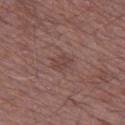This lesion was catalogued during total-body skin photography and was not selected for biopsy. A male patient roughly 50 years of age. The lesion is on the left thigh. An algorithmic analysis of the crop reported an average lesion color of about L≈43 a*≈19 b*≈21 (CIELAB), roughly 6 lightness units darker than nearby skin, and a normalized lesion–skin contrast near 5. The analysis additionally found a classifier nevus-likeness of about 0/100. About 2.5 mm across. Captured under white-light illumination. A roughly 15 mm field-of-view crop from a total-body skin photograph.